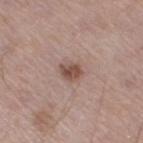The lesion was photographed on a routine skin check and not biopsied; there is no pathology result. The lesion's longest dimension is about 2.5 mm. A male patient aged 53–57. This image is a 15 mm lesion crop taken from a total-body photograph. The lesion is on the right thigh.